Automated tile analysis of the lesion measured an area of roughly 12 mm² and two-axis asymmetry of about 0.25. It also reported a color-variation rating of about 4/10 and a peripheral color-asymmetry measure near 1. And it measured a nevus-likeness score of about 15/100 and a lesion-detection confidence of about 100/100; cropped from a total-body skin-imaging series; the visible field is about 15 mm; from the front of the torso; measured at roughly 5.5 mm in maximum diameter; imaged with white-light lighting; a male subject, aged around 70:
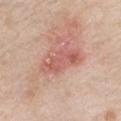Diagnosis: The lesion was biopsied, and histopathology showed a squamous cell carcinoma in situ, classified as a malignant skin lesion.